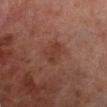| field | value |
|---|---|
| lesion diameter | ~3 mm (longest diameter) |
| TBP lesion metrics | an area of roughly 4.5 mm² and a shape eccentricity near 0.75 |
| site | the left lower leg |
| illumination | cross-polarized |
| image | 15 mm crop, total-body photography |
| patient | male, aged 68 to 72 |